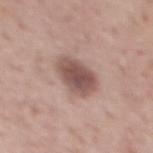Q: Is there a histopathology result?
A: catalogued during a skin exam; not biopsied
Q: Patient demographics?
A: male, aged 68 to 72
Q: Where on the body is the lesion?
A: the mid back
Q: What is the lesion's diameter?
A: ≈4.5 mm
Q: Automated lesion metrics?
A: a detector confidence of about 100 out of 100 that the crop contains a lesion
Q: How was this image acquired?
A: ~15 mm tile from a whole-body skin photo